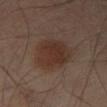Q: Was a biopsy performed?
A: total-body-photography surveillance lesion; no biopsy
Q: What kind of image is this?
A: 15 mm crop, total-body photography
Q: Lesion location?
A: the left thigh
Q: Illumination type?
A: cross-polarized illumination
Q: Patient demographics?
A: male, in their mid- to late 60s
Q: What did automated image analysis measure?
A: a lesion area of about 19 mm², an eccentricity of roughly 0.75, and a symmetry-axis asymmetry near 0.15; a lesion color around L≈30 a*≈16 b*≈22 in CIELAB; a border-irregularity index near 1.5/10, a within-lesion color-variation index near 3/10, and peripheral color asymmetry of about 1